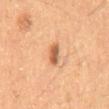Findings:
– imaging modality · total-body-photography crop, ~15 mm field of view
– patient · male, aged 58 to 62